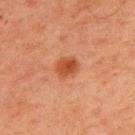The patient is a male about 60 years old.
A 15 mm close-up tile from a total-body photography series done for melanoma screening.
Located on the upper back.